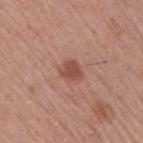<case>
  <biopsy_status>not biopsied; imaged during a skin examination</biopsy_status>
  <lesion_size>
    <long_diameter_mm_approx>2.5</long_diameter_mm_approx>
  </lesion_size>
  <image>
    <source>total-body photography crop</source>
    <field_of_view_mm>15</field_of_view_mm>
  </image>
  <site>right upper arm</site>
  <patient>
    <sex>male</sex>
    <age_approx>55</age_approx>
  </patient>
</case>